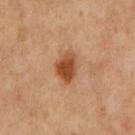follow-up: no biopsy performed (imaged during a skin exam)
body site: the chest
illumination: cross-polarized
subject: male, aged around 65
automated lesion analysis: an eccentricity of roughly 0.7 and a shape-asymmetry score of about 0.25 (0 = symmetric)
acquisition: total-body-photography crop, ~15 mm field of view
lesion diameter: ≈3.5 mm